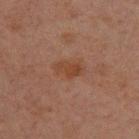follow-up: total-body-photography surveillance lesion; no biopsy
diameter: ~3 mm (longest diameter)
image-analysis metrics: a mean CIELAB color near L≈34 a*≈19 b*≈27 and a lesion-to-skin contrast of about 7 (normalized; higher = more distinct)
subject: male, in their 30s
image source: ~15 mm crop, total-body skin-cancer survey
body site: the upper back